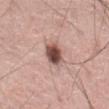Part of a total-body skin-imaging series; this lesion was reviewed on a skin check and was not flagged for biopsy. Imaged with white-light lighting. A male subject, aged 58–62. The total-body-photography lesion software estimated an eccentricity of roughly 0.65. The analysis additionally found a classifier nevus-likeness of about 90/100 and a detector confidence of about 100 out of 100 that the crop contains a lesion. A 15 mm crop from a total-body photograph taken for skin-cancer surveillance. Located on the abdomen.